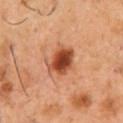Imaged during a routine full-body skin examination; the lesion was not biopsied and no histopathology is available.
Cropped from a whole-body photographic skin survey; the tile spans about 15 mm.
Approximately 4 mm at its widest.
A male patient, roughly 50 years of age.
Imaged with cross-polarized lighting.
On the chest.